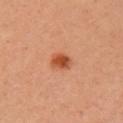workup: imaged on a skin check; not biopsied | tile lighting: cross-polarized illumination | size: ~2.5 mm (longest diameter) | subject: female, aged 33–37 | body site: the arm | imaging modality: 15 mm crop, total-body photography | TBP lesion metrics: a lesion area of about 5 mm² and an outline eccentricity of about 0.6 (0 = round, 1 = elongated); an average lesion color of about L≈51 a*≈30 b*≈38 (CIELAB), about 12 CIELAB-L* units darker than the surrounding skin, and a normalized lesion–skin contrast near 9; an automated nevus-likeness rating near 100 out of 100.